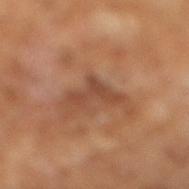Notes:
– notes: total-body-photography surveillance lesion; no biopsy
– tile lighting: cross-polarized
– acquisition: 15 mm crop, total-body photography
– patient: male, aged 63 to 67
– size: ≈4.5 mm